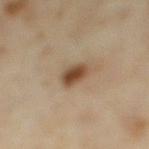biopsy status: catalogued during a skin exam; not biopsied | patient: female, aged 33–37 | anatomic site: the right lower leg | lesion size: about 3 mm | lighting: cross-polarized | imaging modality: ~15 mm crop, total-body skin-cancer survey | automated lesion analysis: an area of roughly 5.5 mm², a shape eccentricity near 0.6, and a shape-asymmetry score of about 0.2 (0 = symmetric); a lesion color around L≈45 a*≈16 b*≈30 in CIELAB, roughly 13 lightness units darker than nearby skin, and a lesion-to-skin contrast of about 10.5 (normalized; higher = more distinct); a border-irregularity index near 2/10 and peripheral color asymmetry of about 1.5.